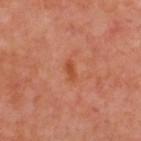biopsy status — total-body-photography surveillance lesion; no biopsy | imaging modality — ~15 mm tile from a whole-body skin photo | automated lesion analysis — a lesion color around L≈49 a*≈29 b*≈37 in CIELAB, about 7 CIELAB-L* units darker than the surrounding skin, and a normalized border contrast of about 7; a nevus-likeness score of about 0/100 and a detector confidence of about 100 out of 100 that the crop contains a lesion | anatomic site — the upper back | lighting — cross-polarized illumination | patient — male, approximately 50 years of age.